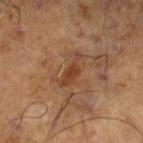Clinical impression: The lesion was tiled from a total-body skin photograph and was not biopsied. Background: An algorithmic analysis of the crop reported a mean CIELAB color near L≈33 a*≈18 b*≈28, about 7 CIELAB-L* units darker than the surrounding skin, and a normalized border contrast of about 7. The analysis additionally found a classifier nevus-likeness of about 0/100 and a detector confidence of about 100 out of 100 that the crop contains a lesion. Located on the right lower leg. Approximately 4 mm at its widest. Cropped from a total-body skin-imaging series; the visible field is about 15 mm. Captured under cross-polarized illumination. A male patient aged approximately 70.